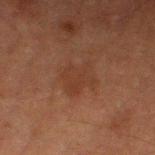notes: total-body-photography surveillance lesion; no biopsy
site: the right lower leg
acquisition: ~15 mm tile from a whole-body skin photo
lesion diameter: ≈3.5 mm
subject: male, roughly 65 years of age
tile lighting: cross-polarized illumination